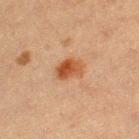Q: Is there a histopathology result?
A: catalogued during a skin exam; not biopsied
Q: Who is the patient?
A: female, aged around 20
Q: Lesion location?
A: the right lower leg
Q: How large is the lesion?
A: ≈3 mm
Q: What did automated image analysis measure?
A: an average lesion color of about L≈47 a*≈24 b*≈36 (CIELAB), about 11 CIELAB-L* units darker than the surrounding skin, and a lesion-to-skin contrast of about 9.5 (normalized; higher = more distinct)
Q: What kind of image is this?
A: ~15 mm crop, total-body skin-cancer survey
Q: Illumination type?
A: cross-polarized